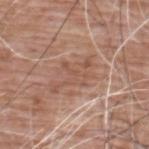Captured during whole-body skin photography for melanoma surveillance; the lesion was not biopsied. A male subject, approximately 60 years of age. Cropped from a total-body skin-imaging series; the visible field is about 15 mm. The lesion is on the upper back.